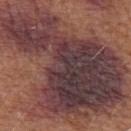Notes:
– follow-up · imaged on a skin check; not biopsied
– subject · male, about 65 years old
– image · total-body-photography crop, ~15 mm field of view
– tile lighting · white-light illumination
– lesion diameter · about 18.5 mm
– anatomic site · the left upper arm